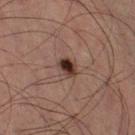Clinical impression: Part of a total-body skin-imaging series; this lesion was reviewed on a skin check and was not flagged for biopsy.